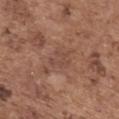Case summary:
- workup — catalogued during a skin exam; not biopsied